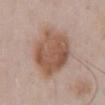notes — catalogued during a skin exam; not biopsied
imaging modality — ~15 mm tile from a whole-body skin photo
diameter — about 7 mm
patient — male, in their mid-50s
anatomic site — the chest
image-analysis metrics — an eccentricity of roughly 0.5 and a symmetry-axis asymmetry near 0.15; roughly 12 lightness units darker than nearby skin and a normalized border contrast of about 9; a nevus-likeness score of about 75/100 and a detector confidence of about 100 out of 100 that the crop contains a lesion
tile lighting — white-light